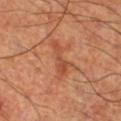This is a cross-polarized tile. Measured at roughly 5 mm in maximum diameter. A 15 mm close-up extracted from a 3D total-body photography capture. Located on the right lower leg. An algorithmic analysis of the crop reported an eccentricity of roughly 0.95 and two-axis asymmetry of about 0.4. A male subject, aged 63 to 67.